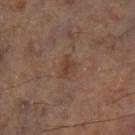Assessment: The lesion was photographed on a routine skin check and not biopsied; there is no pathology result. Image and clinical context: A lesion tile, about 15 mm wide, cut from a 3D total-body photograph. The lesion is located on the left lower leg.